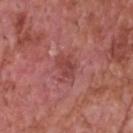- follow-up — catalogued during a skin exam; not biopsied
- TBP lesion metrics — a border-irregularity index near 3/10, internal color variation of about 2 on a 0–10 scale, and radial color variation of about 0.5; a nevus-likeness score of about 0/100 and a detector confidence of about 100 out of 100 that the crop contains a lesion
- lesion size — ~3 mm (longest diameter)
- subject — male, in their 60s
- body site — the head or neck
- image source — ~15 mm crop, total-body skin-cancer survey